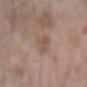Recorded during total-body skin imaging; not selected for excision or biopsy. The lesion is on the left forearm. A female patient, approximately 70 years of age. Measured at roughly 3 mm in maximum diameter. The total-body-photography lesion software estimated a mean CIELAB color near L≈52 a*≈19 b*≈26 and a lesion–skin lightness drop of about 7. A roughly 15 mm field-of-view crop from a total-body skin photograph.